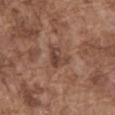Notes:
* workup · total-body-photography surveillance lesion; no biopsy
* image-analysis metrics · a footprint of about 5.5 mm², an eccentricity of roughly 0.65, and two-axis asymmetry of about 0.5; a lesion color around L≈43 a*≈19 b*≈25 in CIELAB and about 9 CIELAB-L* units darker than the surrounding skin; border irregularity of about 5 on a 0–10 scale, a within-lesion color-variation index near 4.5/10, and radial color variation of about 2; an automated nevus-likeness rating near 0 out of 100
* tile lighting · white-light illumination
* diameter · ≈3.5 mm
* site · the abdomen
* image · ~15 mm tile from a whole-body skin photo
* patient · male, in their mid-70s